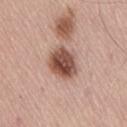Clinical impression:
Part of a total-body skin-imaging series; this lesion was reviewed on a skin check and was not flagged for biopsy.
Acquisition and patient details:
From the back. The total-body-photography lesion software estimated an eccentricity of roughly 0.65 and two-axis asymmetry of about 0.2. And it measured border irregularity of about 2 on a 0–10 scale. The software also gave an automated nevus-likeness rating near 90 out of 100 and a lesion-detection confidence of about 100/100. Imaged with white-light lighting. A region of skin cropped from a whole-body photographic capture, roughly 15 mm wide. A male patient about 55 years old.